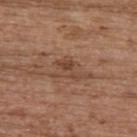Findings:
• notes: total-body-photography surveillance lesion; no biopsy
• image: ~15 mm crop, total-body skin-cancer survey
• subject: female, in their mid- to late 60s
• illumination: white-light illumination
• body site: the upper back